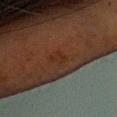Part of a total-body skin-imaging series; this lesion was reviewed on a skin check and was not flagged for biopsy. From the head or neck. Captured under cross-polarized illumination. A 15 mm close-up tile from a total-body photography series done for melanoma screening. The lesion's longest dimension is about 2.5 mm. A female subject, in their 50s.